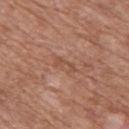Notes:
• biopsy status · total-body-photography surveillance lesion; no biopsy
• subject · male, roughly 60 years of age
• anatomic site · the chest
• acquisition · ~15 mm tile from a whole-body skin photo
• lesion size · about 2.5 mm